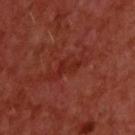Image and clinical context:
A male patient aged 58 to 62. The recorded lesion diameter is about 3 mm. The lesion is located on the upper back. This is a cross-polarized tile. A 15 mm close-up tile from a total-body photography series done for melanoma screening.